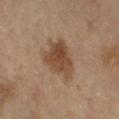No biopsy was performed on this lesion — it was imaged during a full skin examination and was not determined to be concerning.
The lesion is on the right forearm.
Imaged with cross-polarized lighting.
A female patient, approximately 60 years of age.
A 15 mm close-up extracted from a 3D total-body photography capture.
Longest diameter approximately 4.5 mm.